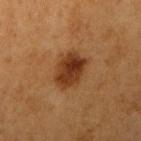Clinical impression: The lesion was tiled from a total-body skin photograph and was not biopsied. Image and clinical context: A 15 mm crop from a total-body photograph taken for skin-cancer surveillance. The tile uses cross-polarized illumination. A male subject aged 58 to 62. The lesion is on the right upper arm.